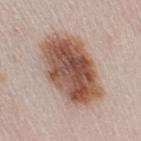anatomic site: the right upper arm | acquisition: ~15 mm tile from a whole-body skin photo | subject: female, aged 48–52 | tile lighting: white-light.